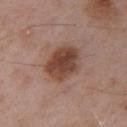No biopsy was performed on this lesion — it was imaged during a full skin examination and was not determined to be concerning. A male subject aged 53–57. This is a white-light tile. Automated tile analysis of the lesion measured a footprint of about 14 mm², an eccentricity of roughly 0.65, and two-axis asymmetry of about 0.15. The analysis additionally found an average lesion color of about L≈43 a*≈21 b*≈27 (CIELAB), roughly 13 lightness units darker than nearby skin, and a lesion-to-skin contrast of about 10 (normalized; higher = more distinct). A 15 mm close-up tile from a total-body photography series done for melanoma screening. The lesion is on the right upper arm.